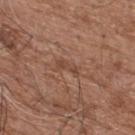follow-up: catalogued during a skin exam; not biopsied | image: ~15 mm tile from a whole-body skin photo | subject: male, in their mid-70s | diameter: ≈3 mm | tile lighting: white-light illumination | body site: the upper back.